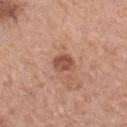Case summary:
- notes · catalogued during a skin exam; not biopsied
- tile lighting · white-light illumination
- patient · female, roughly 60 years of age
- site · the left forearm
- image · 15 mm crop, total-body photography